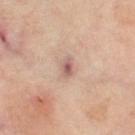{"biopsy_status": "not biopsied; imaged during a skin examination", "automated_metrics": {"cielab_L": 61, "cielab_a": 20, "cielab_b": 24, "vs_skin_darker_L": 11.0, "vs_skin_contrast_norm": 7.0}, "site": "left thigh", "image": {"source": "total-body photography crop", "field_of_view_mm": 15}, "patient": {"sex": "female", "age_approx": 50}, "lighting": "cross-polarized", "lesion_size": {"long_diameter_mm_approx": 2.5}}